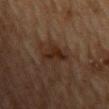workup=imaged on a skin check; not biopsied
location=the mid back
tile lighting=cross-polarized illumination
image=~15 mm crop, total-body skin-cancer survey
subject=male, in their mid-80s or older
diameter=~3 mm (longest diameter)
TBP lesion metrics=an area of roughly 5.5 mm² and a shape eccentricity near 0.4; a nevus-likeness score of about 15/100